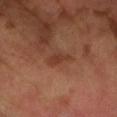Captured during whole-body skin photography for melanoma surveillance; the lesion was not biopsied.
This is a cross-polarized tile.
A lesion tile, about 15 mm wide, cut from a 3D total-body photograph.
The subject is a male in their mid-60s.
About 3 mm across.
The lesion-visualizer software estimated a border-irregularity rating of about 3/10, internal color variation of about 1 on a 0–10 scale, and peripheral color asymmetry of about 0.5. The analysis additionally found a classifier nevus-likeness of about 0/100 and lesion-presence confidence of about 100/100.